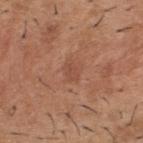biopsy_status: not biopsied; imaged during a skin examination
patient:
  sex: male
  age_approx: 40
site: upper back
automated_metrics:
  cielab_L: 49
  cielab_a: 23
  cielab_b: 31
  vs_skin_darker_L: 6.0
  vs_skin_contrast_norm: 4.5
  nevus_likeness_0_100: 0
  lesion_detection_confidence_0_100: 100
image:
  source: total-body photography crop
  field_of_view_mm: 15